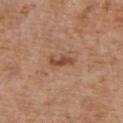{"biopsy_status": "not biopsied; imaged during a skin examination", "lighting": "white-light", "lesion_size": {"long_diameter_mm_approx": 3.5}, "automated_metrics": {"eccentricity": 0.9, "shape_asymmetry": 0.25, "border_irregularity_0_10": 3.0, "color_variation_0_10": 1.0, "peripheral_color_asymmetry": 0.0, "lesion_detection_confidence_0_100": 100}, "image": {"source": "total-body photography crop", "field_of_view_mm": 15}, "patient": {"sex": "female", "age_approx": 75}, "site": "chest"}